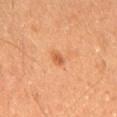Captured during whole-body skin photography for melanoma surveillance; the lesion was not biopsied. A male subject, aged around 60. Automated tile analysis of the lesion measured border irregularity of about 1.5 on a 0–10 scale, internal color variation of about 1.5 on a 0–10 scale, and peripheral color asymmetry of about 0.5. And it measured a nevus-likeness score of about 55/100 and a detector confidence of about 100 out of 100 that the crop contains a lesion. This is a cross-polarized tile. A region of skin cropped from a whole-body photographic capture, roughly 15 mm wide. Longest diameter approximately 2 mm. The lesion is on the mid back.